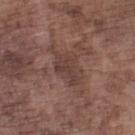biopsy_status: not biopsied; imaged during a skin examination
image:
  source: total-body photography crop
  field_of_view_mm: 15
lighting: white-light
automated_metrics:
  eccentricity: 0.85
  shape_asymmetry: 0.4
  cielab_L: 40
  cielab_a: 18
  cielab_b: 21
  vs_skin_darker_L: 7.0
  vs_skin_contrast_norm: 6.5
  color_variation_0_10: 2.5
  peripheral_color_asymmetry: 1.0
patient:
  sex: male
  age_approx: 75
site: left lower leg